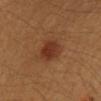follow-up: imaged on a skin check; not biopsied
site: the right upper arm
lesion diameter: ≈3.5 mm
illumination: cross-polarized
subject: male, roughly 40 years of age
image: 15 mm crop, total-body photography
image-analysis metrics: a footprint of about 7.5 mm², a shape eccentricity near 0.55, and a shape-asymmetry score of about 0.15 (0 = symmetric); a lesion-detection confidence of about 100/100